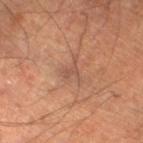Findings:
– workup · imaged on a skin check; not biopsied
– site · the left lower leg
– subject · male, aged around 60
– image · 15 mm crop, total-body photography